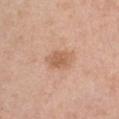{
  "biopsy_status": "not biopsied; imaged during a skin examination",
  "lesion_size": {
    "long_diameter_mm_approx": 3.5
  },
  "patient": {
    "sex": "female",
    "age_approx": 50
  },
  "site": "chest",
  "image": {
    "source": "total-body photography crop",
    "field_of_view_mm": 15
  }
}